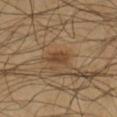notes = total-body-photography surveillance lesion; no biopsy | image = ~15 mm tile from a whole-body skin photo | image-analysis metrics = about 8 CIELAB-L* units darker than the surrounding skin and a normalized border contrast of about 7; a peripheral color-asymmetry measure near 1 | patient = male, roughly 65 years of age | body site = the right lower leg.